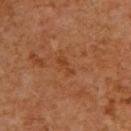  lesion_size:
    long_diameter_mm_approx: 3.0
  image:
    source: total-body photography crop
    field_of_view_mm: 15
  site: upper back
  automated_metrics:
    peripheral_color_asymmetry: 0.0
  patient:
    sex: male
    age_approx: 60